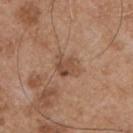Clinical impression:
Part of a total-body skin-imaging series; this lesion was reviewed on a skin check and was not flagged for biopsy.
Background:
Imaged with white-light lighting. The lesion is on the chest. A male subject in their mid- to late 50s. Cropped from a whole-body photographic skin survey; the tile spans about 15 mm.